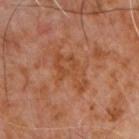biopsy_status: not biopsied; imaged during a skin examination
site: chest
lesion_size:
  long_diameter_mm_approx: 6.0
lighting: cross-polarized
patient:
  sex: male
  age_approx: 60
image:
  source: total-body photography crop
  field_of_view_mm: 15